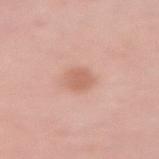Impression:
Recorded during total-body skin imaging; not selected for excision or biopsy.
Clinical summary:
Located on the left upper arm. Longest diameter approximately 3 mm. A female subject roughly 50 years of age. The lesion-visualizer software estimated a footprint of about 5.5 mm², an outline eccentricity of about 0.7 (0 = round, 1 = elongated), and a symmetry-axis asymmetry near 0.2. It also reported an automated nevus-likeness rating near 55 out of 100 and a lesion-detection confidence of about 100/100. A 15 mm crop from a total-body photograph taken for skin-cancer surveillance.